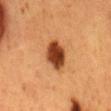<lesion>
<image>
  <source>total-body photography crop</source>
  <field_of_view_mm>15</field_of_view_mm>
</image>
<site>back</site>
<patient>
  <sex>female</sex>
  <age_approx>50</age_approx>
</patient>
</lesion>